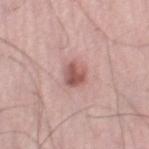Assessment:
No biopsy was performed on this lesion — it was imaged during a full skin examination and was not determined to be concerning.
Clinical summary:
Located on the leg. The patient is a male roughly 50 years of age. A 15 mm crop from a total-body photograph taken for skin-cancer surveillance.